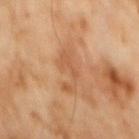image:
  source: total-body photography crop
  field_of_view_mm: 15
patient:
  sex: male
  age_approx: 60
automated_metrics:
  area_mm2_approx: 8.5
  eccentricity: 0.9
  shape_asymmetry: 0.45
  vs_skin_darker_L: 7.0
  vs_skin_contrast_norm: 5.0
  border_irregularity_0_10: 7.0
  color_variation_0_10: 2.0
  nevus_likeness_0_100: 0
  lesion_detection_confidence_0_100: 100
lighting: cross-polarized
site: abdomen
lesion_size:
  long_diameter_mm_approx: 5.5